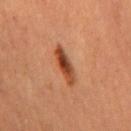Findings:
• follow-up — imaged on a skin check; not biopsied
• TBP lesion metrics — an average lesion color of about L≈40 a*≈25 b*≈33 (CIELAB), roughly 13 lightness units darker than nearby skin, and a normalized border contrast of about 10.5; a border-irregularity index near 3/10 and internal color variation of about 6.5 on a 0–10 scale
• size — ≈4.5 mm
• image source — ~15 mm crop, total-body skin-cancer survey
• body site — the mid back
• patient — male, aged around 65
• tile lighting — cross-polarized illumination